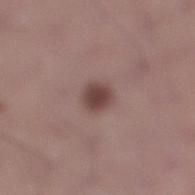  biopsy_status: not biopsied; imaged during a skin examination
  lesion_size:
    long_diameter_mm_approx: 2.5
  lighting: white-light
  automated_metrics:
    nevus_likeness_0_100: 95
    lesion_detection_confidence_0_100: 100
  image:
    source: total-body photography crop
    field_of_view_mm: 15
  site: right lower leg
  patient:
    sex: male
    age_approx: 35